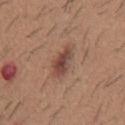biopsy status: total-body-photography surveillance lesion; no biopsy | image: 15 mm crop, total-body photography | size: about 3.5 mm | lighting: white-light | subject: male, in their 60s | body site: the mid back.